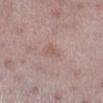Notes:
* follow-up · imaged on a skin check; not biopsied
* tile lighting · white-light
* location · the right lower leg
* patient · female, aged 43–47
* image · 15 mm crop, total-body photography
* diameter · ≈3 mm